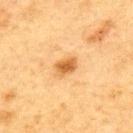The lesion is on the upper back.
The patient is a male in their mid- to late 70s.
Automated tile analysis of the lesion measured a shape eccentricity near 0.75. The software also gave a lesion color around L≈50 a*≈21 b*≈40 in CIELAB, roughly 12 lightness units darker than nearby skin, and a lesion-to-skin contrast of about 8.5 (normalized; higher = more distinct). The software also gave border irregularity of about 2 on a 0–10 scale, internal color variation of about 2.5 on a 0–10 scale, and radial color variation of about 0.5.
Imaged with cross-polarized lighting.
A 15 mm crop from a total-body photograph taken for skin-cancer surveillance.